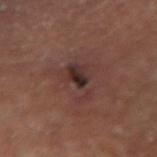Clinical impression:
Recorded during total-body skin imaging; not selected for excision or biopsy.
Clinical summary:
The lesion is located on the arm. The tile uses cross-polarized illumination. A lesion tile, about 15 mm wide, cut from a 3D total-body photograph. A male subject, roughly 65 years of age. About 4.5 mm across.